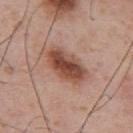{
  "biopsy_status": "not biopsied; imaged during a skin examination"
}